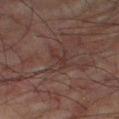| feature | finding |
|---|---|
| biopsy status | no biopsy performed (imaged during a skin exam) |
| diameter | ≈4 mm |
| subject | male, aged 73–77 |
| image | 15 mm crop, total-body photography |
| body site | the right thigh |
| image-analysis metrics | border irregularity of about 6 on a 0–10 scale and radial color variation of about 0.5; an automated nevus-likeness rating near 0 out of 100 |
| lighting | cross-polarized illumination |